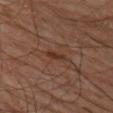workup: no biopsy performed (imaged during a skin exam); subject: male, in their mid- to late 60s; size: about 3.5 mm; anatomic site: the left thigh; acquisition: total-body-photography crop, ~15 mm field of view; automated metrics: a nevus-likeness score of about 5/100 and lesion-presence confidence of about 100/100; tile lighting: cross-polarized illumination.